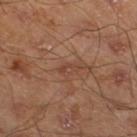Clinical impression: The lesion was tiled from a total-body skin photograph and was not biopsied. Clinical summary: The tile uses cross-polarized illumination. Approximately 2.5 mm at its widest. On the left lower leg. A male patient, aged 43–47. A region of skin cropped from a whole-body photographic capture, roughly 15 mm wide.